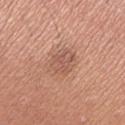The lesion was tiled from a total-body skin photograph and was not biopsied. On the right upper arm. An algorithmic analysis of the crop reported a lesion color around L≈56 a*≈22 b*≈29 in CIELAB and a lesion–skin lightness drop of about 8. The analysis additionally found a classifier nevus-likeness of about 10/100 and a detector confidence of about 100 out of 100 that the crop contains a lesion. This is a white-light tile. The recorded lesion diameter is about 3.5 mm. A roughly 15 mm field-of-view crop from a total-body skin photograph. A female subject, aged 23 to 27.